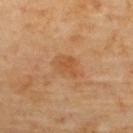follow-up: total-body-photography surveillance lesion; no biopsy
site: the upper back
image-analysis metrics: an average lesion color of about L≈54 a*≈24 b*≈40 (CIELAB) and a normalized border contrast of about 5.5; a border-irregularity rating of about 3/10, a color-variation rating of about 2.5/10, and radial color variation of about 1; an automated nevus-likeness rating near 20 out of 100
acquisition: total-body-photography crop, ~15 mm field of view
patient: female, aged around 60